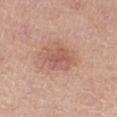  biopsy_status: not biopsied; imaged during a skin examination
  image:
    source: total-body photography crop
    field_of_view_mm: 15
  lesion_size:
    long_diameter_mm_approx: 4.0
  lighting: white-light
  site: leg
  automated_metrics:
    eccentricity: 0.65
    shape_asymmetry: 0.25
  patient:
    sex: female
    age_approx: 65